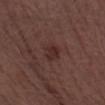| feature | finding |
|---|---|
| biopsy status | no biopsy performed (imaged during a skin exam) |
| subject | male, about 70 years old |
| body site | the left thigh |
| image | ~15 mm crop, total-body skin-cancer survey |